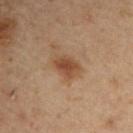The total-body-photography lesion software estimated an outline eccentricity of about 0.75 (0 = round, 1 = elongated) and a symmetry-axis asymmetry near 0.2. The analysis additionally found a lesion color around L≈47 a*≈19 b*≈32 in CIELAB, about 10 CIELAB-L* units darker than the surrounding skin, and a normalized lesion–skin contrast near 8. It also reported a border-irregularity index near 2/10 and internal color variation of about 3.5 on a 0–10 scale. And it measured a classifier nevus-likeness of about 85/100 and lesion-presence confidence of about 100/100.
This is a cross-polarized tile.
Located on the right upper arm.
The subject is a male aged approximately 55.
The recorded lesion diameter is about 4 mm.
A lesion tile, about 15 mm wide, cut from a 3D total-body photograph.